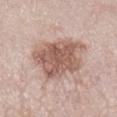  biopsy_status: not biopsied; imaged during a skin examination
  lighting: white-light
  patient:
    sex: female
    age_approx: 65
  lesion_size:
    long_diameter_mm_approx: 7.0
  site: right lower leg
  image:
    source: total-body photography crop
    field_of_view_mm: 15
  automated_metrics:
    cielab_L: 58
    cielab_a: 19
    cielab_b: 25
    vs_skin_darker_L: 13.0
    vs_skin_contrast_norm: 8.5
    nevus_likeness_0_100: 20